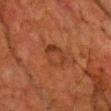Captured during whole-body skin photography for melanoma surveillance; the lesion was not biopsied.
The lesion is on the chest.
Imaged with cross-polarized lighting.
Longest diameter approximately 3.5 mm.
An algorithmic analysis of the crop reported an area of roughly 6.5 mm² and a shape-asymmetry score of about 0.2 (0 = symmetric). It also reported a mean CIELAB color near L≈31 a*≈22 b*≈29 and a normalized lesion–skin contrast near 5.5. And it measured a border-irregularity index near 2/10 and a within-lesion color-variation index near 4.5/10.
The patient is a male aged 63 to 67.
This image is a 15 mm lesion crop taken from a total-body photograph.